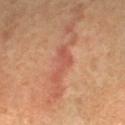The patient is a female about 60 years old. The lesion-visualizer software estimated an area of roughly 5 mm² and two-axis asymmetry of about 0.65. The software also gave an average lesion color of about L≈50 a*≈27 b*≈30 (CIELAB), a lesion–skin lightness drop of about 7, and a normalized border contrast of about 5.5. A region of skin cropped from a whole-body photographic capture, roughly 15 mm wide. Imaged with cross-polarized lighting. About 4.5 mm across. The lesion is located on the right forearm.